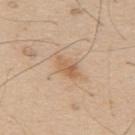Q: Was this lesion biopsied?
A: total-body-photography surveillance lesion; no biopsy
Q: What lighting was used for the tile?
A: white-light
Q: What are the patient's age and sex?
A: male, in their 60s
Q: What kind of image is this?
A: 15 mm crop, total-body photography
Q: Lesion location?
A: the upper back
Q: Lesion size?
A: ≈3.5 mm